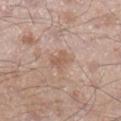{"biopsy_status": "not biopsied; imaged during a skin examination", "image": {"source": "total-body photography crop", "field_of_view_mm": 15}, "site": "right lower leg", "automated_metrics": {"eccentricity": 0.75, "border_irregularity_0_10": 3.5, "color_variation_0_10": 1.5, "peripheral_color_asymmetry": 0.5}, "patient": {"sex": "male", "age_approx": 35}, "lighting": "white-light"}